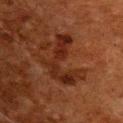follow-up=no biopsy performed (imaged during a skin exam) | lighting=cross-polarized illumination | image=~15 mm tile from a whole-body skin photo | patient=male, approximately 80 years of age | location=the chest.